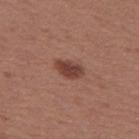Assessment: The lesion was photographed on a routine skin check and not biopsied; there is no pathology result. Clinical summary: A female subject roughly 40 years of age. Located on the left thigh. Approximately 3.5 mm at its widest. A roughly 15 mm field-of-view crop from a total-body skin photograph.